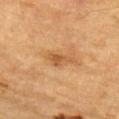Impression:
The lesion was tiled from a total-body skin photograph and was not biopsied.